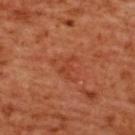No biopsy was performed on this lesion — it was imaged during a full skin examination and was not determined to be concerning.